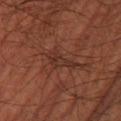<case>
<automated_metrics>
  <area_mm2_approx>5.5</area_mm2_approx>
  <eccentricity>0.9</eccentricity>
  <shape_asymmetry>0.4</shape_asymmetry>
  <vs_skin_darker_L>5.0</vs_skin_darker_L>
  <lesion_detection_confidence_0_100>65</lesion_detection_confidence_0_100>
</automated_metrics>
<site>leg</site>
<lesion_size>
  <long_diameter_mm_approx>4.0</long_diameter_mm_approx>
</lesion_size>
<lighting>cross-polarized</lighting>
<patient>
  <sex>male</sex>
  <age_approx>50</age_approx>
</patient>
<image>
  <source>total-body photography crop</source>
  <field_of_view_mm>15</field_of_view_mm>
</image>
</case>